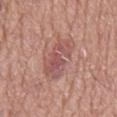  biopsy_status: not biopsied; imaged during a skin examination
  image:
    source: total-body photography crop
    field_of_view_mm: 15
  patient:
    sex: male
    age_approx: 75
  site: mid back
  lighting: white-light